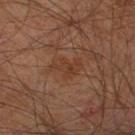{"biopsy_status": "not biopsied; imaged during a skin examination", "lighting": "cross-polarized", "image": {"source": "total-body photography crop", "field_of_view_mm": 15}, "patient": {"sex": "male", "age_approx": 60}, "site": "right leg"}